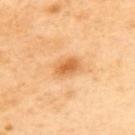Case summary:
• biopsy status: no biopsy performed (imaged during a skin exam)
• subject: female, roughly 40 years of age
• illumination: cross-polarized illumination
• site: the upper back
• automated metrics: a footprint of about 5 mm² and two-axis asymmetry of about 0.15; a border-irregularity index near 1.5/10 and radial color variation of about 0.5; an automated nevus-likeness rating near 75 out of 100
• image: 15 mm crop, total-body photography
• diameter: ~3 mm (longest diameter)